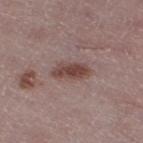The lesion was photographed on a routine skin check and not biopsied; there is no pathology result.
A female patient in their mid- to late 40s.
Imaged with white-light lighting.
The lesion is on the left lower leg.
About 4 mm across.
This image is a 15 mm lesion crop taken from a total-body photograph.
The total-body-photography lesion software estimated an area of roughly 6.5 mm², a shape eccentricity near 0.9, and a shape-asymmetry score of about 0.25 (0 = symmetric). And it measured a mean CIELAB color near L≈44 a*≈19 b*≈21, roughly 11 lightness units darker than nearby skin, and a normalized border contrast of about 9. And it measured a border-irregularity index near 3/10 and peripheral color asymmetry of about 1.5. It also reported an automated nevus-likeness rating near 75 out of 100 and a lesion-detection confidence of about 100/100.